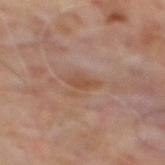– workup · total-body-photography surveillance lesion; no biopsy
– diameter · ≈3 mm
– image · total-body-photography crop, ~15 mm field of view
– location · the mid back
– patient · male, in their mid- to late 60s